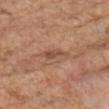Findings:
* follow-up: total-body-photography surveillance lesion; no biopsy
* patient: male, aged approximately 80
* image source: 15 mm crop, total-body photography
* body site: the chest
* automated metrics: an area of roughly 4 mm², an outline eccentricity of about 0.9 (0 = round, 1 = elongated), and a shape-asymmetry score of about 0.35 (0 = symmetric)
* lesion size: ~3.5 mm (longest diameter)
* lighting: white-light illumination